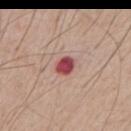{"biopsy_status": "not biopsied; imaged during a skin examination", "lighting": "white-light", "patient": {"sex": "male", "age_approx": 55}, "image": {"source": "total-body photography crop", "field_of_view_mm": 15}, "lesion_size": {"long_diameter_mm_approx": 2.5}}